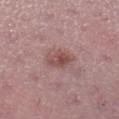Captured during whole-body skin photography for melanoma surveillance; the lesion was not biopsied.
The lesion-visualizer software estimated a lesion–skin lightness drop of about 9 and a normalized lesion–skin contrast near 7. It also reported a nevus-likeness score of about 60/100 and lesion-presence confidence of about 100/100.
The subject is a female in their mid-40s.
Imaged with white-light lighting.
From the left lower leg.
The recorded lesion diameter is about 4 mm.
A 15 mm close-up tile from a total-body photography series done for melanoma screening.